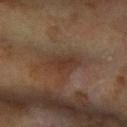<case>
<biopsy_status>not biopsied; imaged during a skin examination</biopsy_status>
<lesion_size>
  <long_diameter_mm_approx>4.5</long_diameter_mm_approx>
</lesion_size>
<patient>
  <sex>female</sex>
  <age_approx>60</age_approx>
</patient>
<site>right forearm</site>
<image>
  <source>total-body photography crop</source>
  <field_of_view_mm>15</field_of_view_mm>
</image>
<lighting>cross-polarized</lighting>
</case>